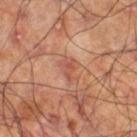notes=catalogued during a skin exam; not biopsied
location=the leg
lesion size=about 3 mm
automated metrics=an automated nevus-likeness rating near 0 out of 100 and a detector confidence of about 100 out of 100 that the crop contains a lesion
subject=male, aged around 60
image source=total-body-photography crop, ~15 mm field of view